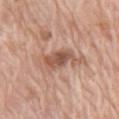Assessment: The lesion was photographed on a routine skin check and not biopsied; there is no pathology result. Acquisition and patient details: On the arm. Cropped from a whole-body photographic skin survey; the tile spans about 15 mm. Longest diameter approximately 4 mm. The subject is a female approximately 75 years of age. Automated tile analysis of the lesion measured about 11 CIELAB-L* units darker than the surrounding skin and a normalized lesion–skin contrast near 7.5. It also reported a nevus-likeness score of about 0/100 and a detector confidence of about 100 out of 100 that the crop contains a lesion. Captured under white-light illumination.